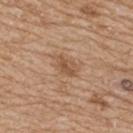<case>
<biopsy_status>not biopsied; imaged during a skin examination</biopsy_status>
<image>
  <source>total-body photography crop</source>
  <field_of_view_mm>15</field_of_view_mm>
</image>
<site>back</site>
<automated_metrics>
  <area_mm2_approx>4.0</area_mm2_approx>
  <eccentricity>0.8</eccentricity>
  <shape_asymmetry>0.3</shape_asymmetry>
  <cielab_L>52</cielab_L>
  <cielab_a>19</cielab_a>
  <cielab_b>33</cielab_b>
  <vs_skin_darker_L>9.0</vs_skin_darker_L>
  <vs_skin_contrast_norm>6.5</vs_skin_contrast_norm>
  <nevus_likeness_0_100>5</nevus_likeness_0_100>
  <lesion_detection_confidence_0_100>100</lesion_detection_confidence_0_100>
</automated_metrics>
<lesion_size>
  <long_diameter_mm_approx>3.0</long_diameter_mm_approx>
</lesion_size>
<patient>
  <sex>female</sex>
  <age_approx>70</age_approx>
</patient>
<lighting>white-light</lighting>
</case>